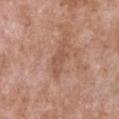The lesion was tiled from a total-body skin photograph and was not biopsied. A female subject, about 75 years old. The lesion is located on the chest. This image is a 15 mm lesion crop taken from a total-body photograph. Approximately 4.5 mm at its widest.